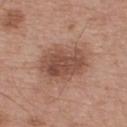{"biopsy_status": "not biopsied; imaged during a skin examination", "lighting": "white-light", "patient": {"sex": "male", "age_approx": 65}, "site": "upper back", "image": {"source": "total-body photography crop", "field_of_view_mm": 15}}